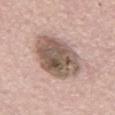Case summary:
• follow-up: catalogued during a skin exam; not biopsied
• image: 15 mm crop, total-body photography
• location: the abdomen
• patient: male, approximately 75 years of age
• image-analysis metrics: a shape eccentricity near 0.7 and two-axis asymmetry of about 0.1; an average lesion color of about L≈55 a*≈15 b*≈23 (CIELAB), roughly 16 lightness units darker than nearby skin, and a normalized border contrast of about 10; a border-irregularity rating of about 1.5/10; a classifier nevus-likeness of about 0/100 and a lesion-detection confidence of about 100/100